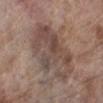| key | value |
|---|---|
| biopsy status | catalogued during a skin exam; not biopsied |
| anatomic site | the left lower leg |
| image-analysis metrics | a lesion color around L≈46 a*≈15 b*≈22 in CIELAB, a lesion–skin lightness drop of about 9, and a lesion-to-skin contrast of about 7 (normalized; higher = more distinct); a border-irregularity index near 7/10, a color-variation rating of about 5.5/10, and radial color variation of about 1.5 |
| subject | female, in their mid- to late 80s or older |
| illumination | white-light illumination |
| lesion size | ~8.5 mm (longest diameter) |
| imaging modality | ~15 mm tile from a whole-body skin photo |